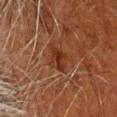Recorded during total-body skin imaging; not selected for excision or biopsy.
Cropped from a whole-body photographic skin survey; the tile spans about 15 mm.
A male patient, aged around 80.
The lesion is located on the head or neck.